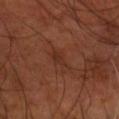Part of a total-body skin-imaging series; this lesion was reviewed on a skin check and was not flagged for biopsy.
The lesion is located on the right forearm.
Cropped from a whole-body photographic skin survey; the tile spans about 15 mm.
A male patient, approximately 55 years of age.
Automated image analysis of the tile measured an average lesion color of about L≈28 a*≈21 b*≈25 (CIELAB), about 4 CIELAB-L* units darker than the surrounding skin, and a normalized border contrast of about 4.5. It also reported border irregularity of about 4 on a 0–10 scale.
Measured at roughly 4 mm in maximum diameter.